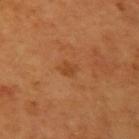Assessment: The lesion was tiled from a total-body skin photograph and was not biopsied. Context: The lesion's longest dimension is about 1.5 mm. A 15 mm close-up extracted from a 3D total-body photography capture. Located on the arm. The patient is a female in their mid- to late 50s. Imaged with cross-polarized lighting.